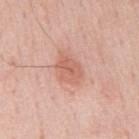workup: imaged on a skin check; not biopsied
patient: male, about 60 years old
location: the mid back
imaging modality: ~15 mm tile from a whole-body skin photo